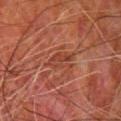Recorded during total-body skin imaging; not selected for excision or biopsy.
The patient is a male aged 63 to 67.
The recorded lesion diameter is about 3 mm.
On the chest.
A roughly 15 mm field-of-view crop from a total-body skin photograph.
Captured under cross-polarized illumination.